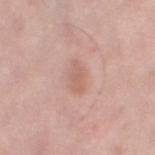A female subject, roughly 50 years of age. The recorded lesion diameter is about 3.5 mm. The tile uses white-light illumination. Located on the left thigh. A 15 mm close-up tile from a total-body photography series done for melanoma screening. The lesion-visualizer software estimated a lesion area of about 4 mm², an outline eccentricity of about 0.9 (0 = round, 1 = elongated), and two-axis asymmetry of about 0.2. And it measured a mean CIELAB color near L≈61 a*≈22 b*≈26, roughly 8 lightness units darker than nearby skin, and a lesion-to-skin contrast of about 5 (normalized; higher = more distinct).